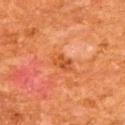| field | value |
|---|---|
| biopsy status | no biopsy performed (imaged during a skin exam) |
| location | the back |
| image source | 15 mm crop, total-body photography |
| illumination | cross-polarized illumination |
| automated lesion analysis | an average lesion color of about L≈52 a*≈33 b*≈44 (CIELAB), about 8 CIELAB-L* units darker than the surrounding skin, and a normalized lesion–skin contrast near 6; a border-irregularity rating of about 2/10, a within-lesion color-variation index near 4.5/10, and radial color variation of about 1.5; a classifier nevus-likeness of about 0/100 and a lesion-detection confidence of about 100/100 |
| patient | male, about 65 years old |
| lesion size | about 3 mm |